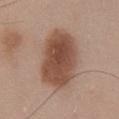Part of a total-body skin-imaging series; this lesion was reviewed on a skin check and was not flagged for biopsy. A male patient aged 53–57. A roughly 15 mm field-of-view crop from a total-body skin photograph. The lesion's longest dimension is about 8.5 mm. Located on the chest. The total-body-photography lesion software estimated a lesion area of about 27 mm², an outline eccentricity of about 0.9 (0 = round, 1 = elongated), and a shape-asymmetry score of about 0.15 (0 = symmetric). And it measured a mean CIELAB color near L≈49 a*≈20 b*≈28 and a normalized lesion–skin contrast near 10. The analysis additionally found a nevus-likeness score of about 100/100 and lesion-presence confidence of about 100/100. Captured under white-light illumination.